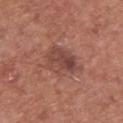  biopsy_status: not biopsied; imaged during a skin examination
  lesion_size:
    long_diameter_mm_approx: 5.0
  site: front of the torso
  lighting: white-light
  patient:
    sex: male
    age_approx: 75
  automated_metrics:
    area_mm2_approx: 13.0
    shape_asymmetry: 0.25
    cielab_L: 46
    cielab_a: 23
    cielab_b: 25
    vs_skin_darker_L: 9.0
    border_irregularity_0_10: 3.0
    color_variation_0_10: 5.0
    nevus_likeness_0_100: 10
    lesion_detection_confidence_0_100: 100
  image:
    source: total-body photography crop
    field_of_view_mm: 15